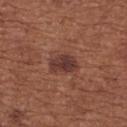notes — total-body-photography surveillance lesion; no biopsy | image-analysis metrics — a lesion area of about 10 mm² and an eccentricity of roughly 0.75; an average lesion color of about L≈39 a*≈21 b*≈24 (CIELAB), a lesion–skin lightness drop of about 8, and a lesion-to-skin contrast of about 7.5 (normalized; higher = more distinct); radial color variation of about 1.5 | tile lighting — white-light | lesion size — ~4 mm (longest diameter) | image source — total-body-photography crop, ~15 mm field of view | patient — female, aged 63–67 | anatomic site — the upper back.